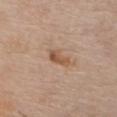This lesion was catalogued during total-body skin photography and was not selected for biopsy.
The patient is a female aged around 70.
Captured under white-light illumination.
The lesion-visualizer software estimated a footprint of about 4 mm², an eccentricity of roughly 0.85, and two-axis asymmetry of about 0.4. It also reported a lesion–skin lightness drop of about 10 and a lesion-to-skin contrast of about 7.5 (normalized; higher = more distinct). It also reported border irregularity of about 4 on a 0–10 scale, a within-lesion color-variation index near 2.5/10, and peripheral color asymmetry of about 0.5. And it measured a nevus-likeness score of about 15/100.
A 15 mm close-up extracted from a 3D total-body photography capture.
On the chest.